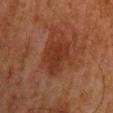Part of a total-body skin-imaging series; this lesion was reviewed on a skin check and was not flagged for biopsy. An algorithmic analysis of the crop reported a lesion area of about 13 mm² and an outline eccentricity of about 0.7 (0 = round, 1 = elongated). It also reported a border-irregularity rating of about 2.5/10 and peripheral color asymmetry of about 0.5. It also reported a nevus-likeness score of about 0/100 and a detector confidence of about 100 out of 100 that the crop contains a lesion. This is a cross-polarized tile. Located on the mid back. A male patient, approximately 80 years of age. The recorded lesion diameter is about 5 mm. A roughly 15 mm field-of-view crop from a total-body skin photograph.